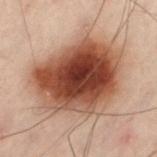biopsy status — catalogued during a skin exam; not biopsied
location — the left thigh
diameter — ~9.5 mm (longest diameter)
subject — male, aged 48 to 52
illumination — cross-polarized illumination
acquisition — ~15 mm crop, total-body skin-cancer survey